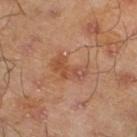<lesion>
  <biopsy_status>not biopsied; imaged during a skin examination</biopsy_status>
  <image>
    <source>total-body photography crop</source>
    <field_of_view_mm>15</field_of_view_mm>
  </image>
  <site>leg</site>
  <lighting>cross-polarized</lighting>
  <patient>
    <sex>male</sex>
    <age_approx>45</age_approx>
  </patient>
  <automated_metrics>
    <area_mm2_approx>7.5</area_mm2_approx>
    <eccentricity>0.85</eccentricity>
    <cielab_L>50</cielab_L>
    <cielab_a>23</cielab_a>
    <cielab_b>33</cielab_b>
    <vs_skin_darker_L>7.0</vs_skin_darker_L>
    <border_irregularity_0_10>5.0</border_irregularity_0_10>
    <color_variation_0_10>5.0</color_variation_0_10>
    <peripheral_color_asymmetry>2.0</peripheral_color_asymmetry>
  </automated_metrics>
</lesion>